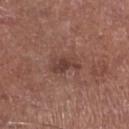No biopsy was performed on this lesion — it was imaged during a full skin examination and was not determined to be concerning.